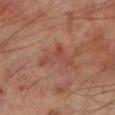illumination = cross-polarized illumination | diameter = ~4.5 mm (longest diameter) | image = total-body-photography crop, ~15 mm field of view | site = the left lower leg | automated lesion analysis = a border-irregularity index near 6.5/10, a color-variation rating of about 4/10, and radial color variation of about 1.5; a nevus-likeness score of about 0/100 and a lesion-detection confidence of about 100/100 | subject = male, aged 68 to 72.